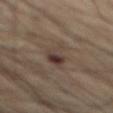Case summary:
- follow-up: total-body-photography surveillance lesion; no biopsy
- patient: male, aged 68 to 72
- anatomic site: the abdomen
- imaging modality: total-body-photography crop, ~15 mm field of view